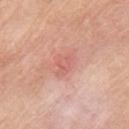{"biopsy_status": "not biopsied; imaged during a skin examination", "lighting": "white-light", "lesion_size": {"long_diameter_mm_approx": 2.0}, "image": {"source": "total-body photography crop", "field_of_view_mm": 15}, "site": "left upper arm", "patient": {"sex": "female", "age_approx": 65}}